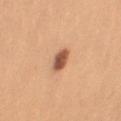Impression: Recorded during total-body skin imaging; not selected for excision or biopsy. Clinical summary: A female patient aged around 25. The lesion's longest dimension is about 2.5 mm. Imaged with white-light lighting. On the right upper arm. A 15 mm close-up tile from a total-body photography series done for melanoma screening.